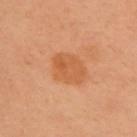Q: Was a biopsy performed?
A: no biopsy performed (imaged during a skin exam)
Q: Who is the patient?
A: female, aged around 60
Q: What is the anatomic site?
A: the chest
Q: Lesion size?
A: about 4.5 mm
Q: Automated lesion metrics?
A: a border-irregularity index near 1.5/10
Q: What lighting was used for the tile?
A: cross-polarized illumination
Q: What kind of image is this?
A: 15 mm crop, total-body photography